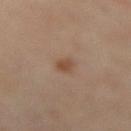biopsy status=total-body-photography surveillance lesion; no biopsy
automated metrics=an area of roughly 3 mm² and two-axis asymmetry of about 0.35; an average lesion color of about L≈45 a*≈18 b*≈28 (CIELAB), roughly 8 lightness units darker than nearby skin, and a normalized border contrast of about 7; a border-irregularity index near 3/10, a color-variation rating of about 1.5/10, and peripheral color asymmetry of about 0.5
patient=male, aged around 65
imaging modality=total-body-photography crop, ~15 mm field of view
lesion diameter=≈2.5 mm
site=the lower back